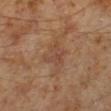Clinical impression:
This lesion was catalogued during total-body skin photography and was not selected for biopsy.
Context:
Cropped from a total-body skin-imaging series; the visible field is about 15 mm. Automated tile analysis of the lesion measured radial color variation of about 0.5. The software also gave an automated nevus-likeness rating near 0 out of 100 and a lesion-detection confidence of about 100/100. From the left lower leg. The tile uses cross-polarized illumination. Approximately 3 mm at its widest. The patient is a male about 60 years old.